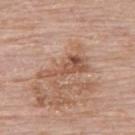follow-up: imaged on a skin check; not biopsied
diameter: ≈6 mm
site: the back
patient: female, aged 63 to 67
image source: total-body-photography crop, ~15 mm field of view